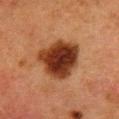Part of a total-body skin-imaging series; this lesion was reviewed on a skin check and was not flagged for biopsy.